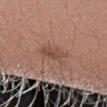Imaged during a routine full-body skin examination; the lesion was not biopsied and no histopathology is available. Cropped from a total-body skin-imaging series; the visible field is about 15 mm. A female patient, aged 23–27. The total-body-photography lesion software estimated a lesion color around L≈48 a*≈20 b*≈26 in CIELAB, about 8 CIELAB-L* units darker than the surrounding skin, and a lesion-to-skin contrast of about 6 (normalized; higher = more distinct). It also reported a border-irregularity rating of about 3/10 and a color-variation rating of about 3/10. On the right forearm. The lesion's longest dimension is about 3.5 mm.